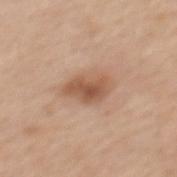Clinical impression:
Recorded during total-body skin imaging; not selected for excision or biopsy.
Image and clinical context:
The lesion-visualizer software estimated a shape eccentricity near 0.8 and a shape-asymmetry score of about 0.2 (0 = symmetric). And it measured border irregularity of about 2.5 on a 0–10 scale, internal color variation of about 4 on a 0–10 scale, and peripheral color asymmetry of about 1.5. It also reported a nevus-likeness score of about 85/100 and a detector confidence of about 100 out of 100 that the crop contains a lesion. The lesion is on the mid back. The subject is a female aged around 65. Cropped from a whole-body photographic skin survey; the tile spans about 15 mm. Measured at roughly 4.5 mm in maximum diameter.